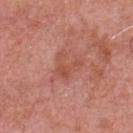The lesion was photographed on a routine skin check and not biopsied; there is no pathology result. Located on the chest. A 15 mm close-up extracted from a 3D total-body photography capture. Automated image analysis of the tile measured a border-irregularity index near 7/10, a within-lesion color-variation index near 2.5/10, and peripheral color asymmetry of about 1. The analysis additionally found an automated nevus-likeness rating near 0 out of 100 and a detector confidence of about 100 out of 100 that the crop contains a lesion. About 3 mm across. Imaged with white-light lighting. The subject is a male approximately 70 years of age.